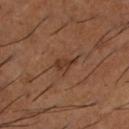The lesion was photographed on a routine skin check and not biopsied; there is no pathology result.
Longest diameter approximately 2.5 mm.
A lesion tile, about 15 mm wide, cut from a 3D total-body photograph.
A male subject in their mid-60s.
The tile uses cross-polarized illumination.
From the leg.